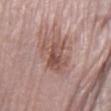No biopsy was performed on this lesion — it was imaged during a full skin examination and was not determined to be concerning.
A roughly 15 mm field-of-view crop from a total-body skin photograph.
A female patient roughly 65 years of age.
Located on the left lower leg.